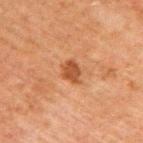Q: Is there a histopathology result?
A: catalogued during a skin exam; not biopsied
Q: What kind of image is this?
A: 15 mm crop, total-body photography
Q: Where on the body is the lesion?
A: the upper back
Q: How was the tile lit?
A: cross-polarized
Q: Who is the patient?
A: male, in their 60s
Q: How large is the lesion?
A: about 2.5 mm